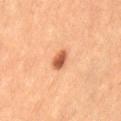Assessment: Part of a total-body skin-imaging series; this lesion was reviewed on a skin check and was not flagged for biopsy. Acquisition and patient details: A female subject, roughly 55 years of age. The lesion is located on the left thigh. The recorded lesion diameter is about 2.5 mm. A 15 mm crop from a total-body photograph taken for skin-cancer surveillance.